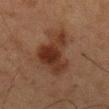<record>
  <biopsy_status>not biopsied; imaged during a skin examination</biopsy_status>
  <image>
    <source>total-body photography crop</source>
    <field_of_view_mm>15</field_of_view_mm>
  </image>
  <lighting>cross-polarized</lighting>
  <lesion_size>
    <long_diameter_mm_approx>7.0</long_diameter_mm_approx>
  </lesion_size>
  <site>mid back</site>
  <patient>
    <sex>male</sex>
    <age_approx>60</age_approx>
  </patient>
</record>